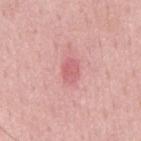workup: catalogued during a skin exam; not biopsied | image-analysis metrics: an eccentricity of roughly 0.7 and a shape-asymmetry score of about 0.25 (0 = symmetric); an average lesion color of about L≈61 a*≈31 b*≈22 (CIELAB); border irregularity of about 2 on a 0–10 scale and internal color variation of about 3.5 on a 0–10 scale; a nevus-likeness score of about 5/100 and a detector confidence of about 100 out of 100 that the crop contains a lesion | body site: the back | lighting: white-light | subject: male, approximately 50 years of age | size: ~2.5 mm (longest diameter) | image: total-body-photography crop, ~15 mm field of view.